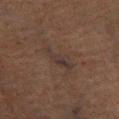Clinical impression: This lesion was catalogued during total-body skin photography and was not selected for biopsy. Background: This is a cross-polarized tile. From the left lower leg. Automated tile analysis of the lesion measured a mean CIELAB color near L≈26 a*≈11 b*≈16, a lesion–skin lightness drop of about 4, and a normalized lesion–skin contrast near 6. The software also gave a nevus-likeness score of about 0/100. A male subject, aged 73 to 77. The recorded lesion diameter is about 5.5 mm. A 15 mm close-up extracted from a 3D total-body photography capture.